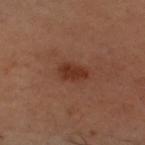workup=total-body-photography surveillance lesion; no biopsy
tile lighting=cross-polarized illumination
imaging modality=total-body-photography crop, ~15 mm field of view
subject=male, in their 30s
diameter=~3.5 mm (longest diameter)
location=the head or neck
image-analysis metrics=an area of roughly 5 mm²; an average lesion color of about L≈26 a*≈19 b*≈24 (CIELAB), about 7 CIELAB-L* units darker than the surrounding skin, and a normalized lesion–skin contrast near 8.5; a border-irregularity index near 2.5/10, internal color variation of about 2 on a 0–10 scale, and a peripheral color-asymmetry measure near 1